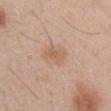Impression:
This lesion was catalogued during total-body skin photography and was not selected for biopsy.
Image and clinical context:
About 2.5 mm across. Located on the right upper arm. A male subject, aged approximately 30. A roughly 15 mm field-of-view crop from a total-body skin photograph.